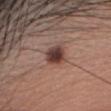– imaging modality — 15 mm crop, total-body photography
– tile lighting — white-light
– diameter — ~2.5 mm (longest diameter)
– subject — female, aged 38–42
– location — the head or neck
– automated lesion analysis — an area of roughly 5.5 mm², an outline eccentricity of about 0.5 (0 = round, 1 = elongated), and two-axis asymmetry of about 0.15; a mean CIELAB color near L≈39 a*≈20 b*≈22 and a lesion–skin lightness drop of about 15; border irregularity of about 1 on a 0–10 scale, a color-variation rating of about 5/10, and peripheral color asymmetry of about 1.5; a detector confidence of about 100 out of 100 that the crop contains a lesion